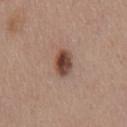workup — total-body-photography surveillance lesion; no biopsy | location — the chest | image — 15 mm crop, total-body photography | size — ≈3.5 mm | subject — male, in their mid-50s | illumination — white-light illumination | image-analysis metrics — an area of roughly 6.5 mm², an eccentricity of roughly 0.8, and two-axis asymmetry of about 0.15; an average lesion color of about L≈45 a*≈20 b*≈26 (CIELAB), roughly 15 lightness units darker than nearby skin, and a normalized lesion–skin contrast near 11.